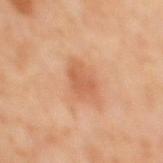Case summary:
– workup · catalogued during a skin exam; not biopsied
– image · ~15 mm tile from a whole-body skin photo
– site · the mid back
– tile lighting · cross-polarized illumination
– automated lesion analysis · a mean CIELAB color near L≈55 a*≈24 b*≈34, about 8 CIELAB-L* units darker than the surrounding skin, and a normalized border contrast of about 5.5; a border-irregularity index near 2.5/10, a within-lesion color-variation index near 1.5/10, and radial color variation of about 0.5
– patient · male, roughly 60 years of age